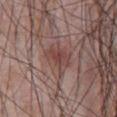A region of skin cropped from a whole-body photographic capture, roughly 15 mm wide. The tile uses white-light illumination. Approximately 4 mm at its widest. A male subject about 65 years old. The lesion is located on the front of the torso.